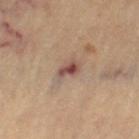{
  "biopsy_status": "not biopsied; imaged during a skin examination",
  "site": "leg",
  "lesion_size": {
    "long_diameter_mm_approx": 3.0
  },
  "lighting": "cross-polarized",
  "patient": {
    "sex": "female",
    "age_approx": 65
  },
  "image": {
    "source": "total-body photography crop",
    "field_of_view_mm": 15
  }
}